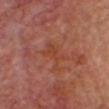Q: Was a biopsy performed?
A: total-body-photography surveillance lesion; no biopsy
Q: What is the lesion's diameter?
A: ≈5 mm
Q: Illumination type?
A: cross-polarized illumination
Q: Where on the body is the lesion?
A: the head or neck
Q: What kind of image is this?
A: 15 mm crop, total-body photography
Q: What are the patient's age and sex?
A: male, in their mid-60s